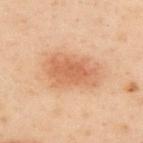biopsy_status: not biopsied; imaged during a skin examination
lighting: cross-polarized
image:
  source: total-body photography crop
  field_of_view_mm: 15
lesion_size:
  long_diameter_mm_approx: 6.0
automated_metrics:
  cielab_L: 51
  cielab_a: 20
  cielab_b: 31
  vs_skin_darker_L: 9.0
  vs_skin_contrast_norm: 6.5
  nevus_likeness_0_100: 100
  lesion_detection_confidence_0_100: 100
patient:
  sex: male
  age_approx: 50
site: upper back